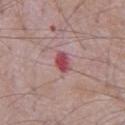<tbp_lesion>
  <biopsy_status>not biopsied; imaged during a skin examination</biopsy_status>
  <image>
    <source>total-body photography crop</source>
    <field_of_view_mm>15</field_of_view_mm>
  </image>
  <lighting>white-light</lighting>
  <patient>
    <sex>male</sex>
    <age_approx>65</age_approx>
  </patient>
  <automated_metrics>
    <cielab_L>48</cielab_L>
    <cielab_a>32</cielab_a>
    <cielab_b>19</cielab_b>
    <vs_skin_darker_L>13.0</vs_skin_darker_L>
    <vs_skin_contrast_norm>9.5</vs_skin_contrast_norm>
    <nevus_likeness_0_100>0</nevus_likeness_0_100>
    <lesion_detection_confidence_0_100>100</lesion_detection_confidence_0_100>
  </automated_metrics>
  <site>chest</site>
  <lesion_size>
    <long_diameter_mm_approx>3.0</long_diameter_mm_approx>
  </lesion_size>
</tbp_lesion>